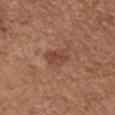The lesion was tiled from a total-body skin photograph and was not biopsied.
The patient is a female about 75 years old.
Automated tile analysis of the lesion measured a lesion color around L≈45 a*≈23 b*≈28 in CIELAB and a lesion–skin lightness drop of about 8. The analysis additionally found internal color variation of about 2 on a 0–10 scale and radial color variation of about 1. The analysis additionally found an automated nevus-likeness rating near 0 out of 100.
A 15 mm crop from a total-body photograph taken for skin-cancer surveillance.
Measured at roughly 3 mm in maximum diameter.
Captured under white-light illumination.
On the chest.